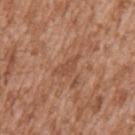Imaged during a routine full-body skin examination; the lesion was not biopsied and no histopathology is available. A male subject, aged 43–47. From the left upper arm. Approximately 4 mm at its widest. This is a white-light tile. A 15 mm close-up extracted from a 3D total-body photography capture.